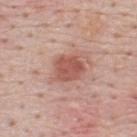biopsy_status: not biopsied; imaged during a skin examination
patient:
  sex: male
  age_approx: 50
site: back
image:
  source: total-body photography crop
  field_of_view_mm: 15
lesion_size:
  long_diameter_mm_approx: 3.5